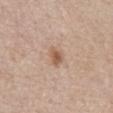The lesion was photographed on a routine skin check and not biopsied; there is no pathology result. The total-body-photography lesion software estimated an area of roughly 3.5 mm², an eccentricity of roughly 0.7, and a shape-asymmetry score of about 0.3 (0 = symmetric). The analysis additionally found a within-lesion color-variation index near 2/10 and a peripheral color-asymmetry measure near 0.5. Longest diameter approximately 2.5 mm. This is a white-light tile. The lesion is located on the abdomen. A 15 mm close-up tile from a total-body photography series done for melanoma screening. The patient is a female in their mid-60s.